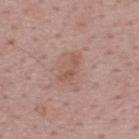The lesion was tiled from a total-body skin photograph and was not biopsied. A male subject aged approximately 50. A lesion tile, about 15 mm wide, cut from a 3D total-body photograph. Located on the upper back.